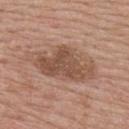Notes:
- follow-up: imaged on a skin check; not biopsied
- lesion diameter: ≈8.5 mm
- patient: female, about 50 years old
- acquisition: ~15 mm tile from a whole-body skin photo
- lighting: white-light illumination
- site: the back